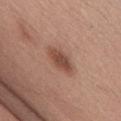biopsy status: imaged on a skin check; not biopsied
automated metrics: a within-lesion color-variation index near 2.5/10
illumination: white-light illumination
site: the abdomen
patient: male, in their mid-50s
acquisition: ~15 mm tile from a whole-body skin photo
lesion diameter: about 4 mm